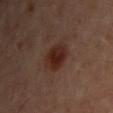Impression:
The lesion was tiled from a total-body skin photograph and was not biopsied.